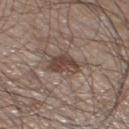Impression:
The lesion was photographed on a routine skin check and not biopsied; there is no pathology result.
Image and clinical context:
The patient is a male about 60 years old. Imaged with white-light lighting. The lesion is located on the leg. Cropped from a total-body skin-imaging series; the visible field is about 15 mm. Longest diameter approximately 4 mm. Automated tile analysis of the lesion measured a border-irregularity index near 4/10, a color-variation rating of about 3.5/10, and a peripheral color-asymmetry measure near 1.5. It also reported a classifier nevus-likeness of about 60/100 and a detector confidence of about 95 out of 100 that the crop contains a lesion.